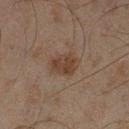Notes:
– biopsy status — no biopsy performed (imaged during a skin exam)
– subject — male, aged 43 to 47
– image source — total-body-photography crop, ~15 mm field of view
– location — the left lower leg